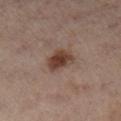| key | value |
|---|---|
| biopsy status | imaged on a skin check; not biopsied |
| illumination | cross-polarized |
| automated metrics | an automated nevus-likeness rating near 100 out of 100 and a lesion-detection confidence of about 100/100 |
| patient | female, in their mid- to late 50s |
| lesion diameter | ~3 mm (longest diameter) |
| location | the left leg |
| image | ~15 mm tile from a whole-body skin photo |